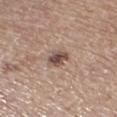Clinical summary: About 2.5 mm across. This is a white-light tile. On the leg. The subject is a female about 75 years old. This image is a 15 mm lesion crop taken from a total-body photograph.